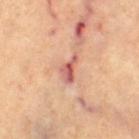Clinical impression: No biopsy was performed on this lesion — it was imaged during a full skin examination and was not determined to be concerning. Context: A female subject, aged 63 to 67. Longest diameter approximately 4 mm. On the left thigh. A roughly 15 mm field-of-view crop from a total-body skin photograph. Automated tile analysis of the lesion measured a border-irregularity rating of about 4.5/10, internal color variation of about 7.5 on a 0–10 scale, and peripheral color asymmetry of about 2.5. The analysis additionally found a classifier nevus-likeness of about 0/100 and lesion-presence confidence of about 100/100. Imaged with cross-polarized lighting.